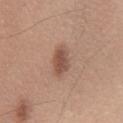<case>
  <biopsy_status>not biopsied; imaged during a skin examination</biopsy_status>
  <patient>
    <sex>male</sex>
    <age_approx>55</age_approx>
  </patient>
  <image>
    <source>total-body photography crop</source>
    <field_of_view_mm>15</field_of_view_mm>
  </image>
  <site>chest</site>
  <lighting>white-light</lighting>
  <lesion_size>
    <long_diameter_mm_approx>4.0</long_diameter_mm_approx>
  </lesion_size>
  <automated_metrics>
    <lesion_detection_confidence_0_100>100</lesion_detection_confidence_0_100>
  </automated_metrics>
</case>